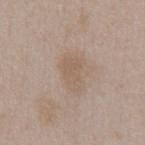Q: Is there a histopathology result?
A: no biopsy performed (imaged during a skin exam)
Q: What is the imaging modality?
A: ~15 mm tile from a whole-body skin photo
Q: Who is the patient?
A: male, in their 50s
Q: Where on the body is the lesion?
A: the front of the torso